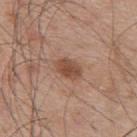workup = catalogued during a skin exam; not biopsied | TBP lesion metrics = a mean CIELAB color near L≈48 a*≈21 b*≈29 and a normalized border contrast of about 8; border irregularity of about 1.5 on a 0–10 scale, a color-variation rating of about 3/10, and radial color variation of about 1 | subject = male, aged 53 to 57 | size = about 3.5 mm | lighting = white-light | acquisition = ~15 mm crop, total-body skin-cancer survey | site = the upper back.